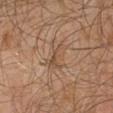{
  "biopsy_status": "not biopsied; imaged during a skin examination",
  "automated_metrics": {
    "area_mm2_approx": 7.0,
    "shape_asymmetry": 0.35,
    "cielab_L": 47,
    "cielab_a": 15,
    "cielab_b": 29,
    "vs_skin_darker_L": 6.0,
    "vs_skin_contrast_norm": 5.0,
    "border_irregularity_0_10": 4.5,
    "color_variation_0_10": 4.0,
    "peripheral_color_asymmetry": 1.5,
    "nevus_likeness_0_100": 0,
    "lesion_detection_confidence_0_100": 75
  },
  "site": "right forearm",
  "lighting": "cross-polarized",
  "image": {
    "source": "total-body photography crop",
    "field_of_view_mm": 15
  },
  "patient": {
    "sex": "male",
    "age_approx": 65
  },
  "lesion_size": {
    "long_diameter_mm_approx": 3.5
  }
}